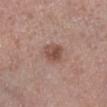{"patient": {"sex": "male", "age_approx": 55}, "image": {"source": "total-body photography crop", "field_of_view_mm": 15}, "site": "right lower leg"}